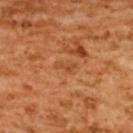Automated tile analysis of the lesion measured an outline eccentricity of about 0.85 (0 = round, 1 = elongated) and two-axis asymmetry of about 0.5. And it measured a classifier nevus-likeness of about 0/100. A female patient aged around 55. This is a cross-polarized tile. Cropped from a total-body skin-imaging series; the visible field is about 15 mm. The lesion is on the upper back. Approximately 2.5 mm at its widest.